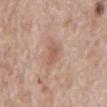No biopsy was performed on this lesion — it was imaged during a full skin examination and was not determined to be concerning.
A male patient, aged around 70.
Imaged with white-light lighting.
A 15 mm close-up tile from a total-body photography series done for melanoma screening.
Located on the front of the torso.